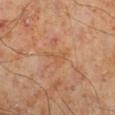{"biopsy_status": "not biopsied; imaged during a skin examination", "site": "leg", "lighting": "cross-polarized", "image": {"source": "total-body photography crop", "field_of_view_mm": 15}, "patient": {"sex": "male", "age_approx": 70}}